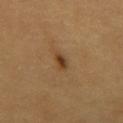Assessment:
Captured during whole-body skin photography for melanoma surveillance; the lesion was not biopsied.
Clinical summary:
Cropped from a total-body skin-imaging series; the visible field is about 15 mm. This is a cross-polarized tile. Measured at roughly 2.5 mm in maximum diameter. A male patient aged approximately 60. The lesion is located on the mid back.